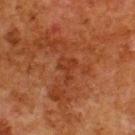biopsy status: no biopsy performed (imaged during a skin exam)
body site: the back
subject: male, about 80 years old
acquisition: ~15 mm tile from a whole-body skin photo
lesion diameter: ~3 mm (longest diameter)